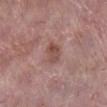Impression: Captured during whole-body skin photography for melanoma surveillance; the lesion was not biopsied. Background: A female patient approximately 60 years of age. The lesion is on the leg. Approximately 3 mm at its widest. A region of skin cropped from a whole-body photographic capture, roughly 15 mm wide. This is a white-light tile.